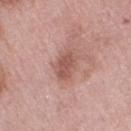| feature | finding |
|---|---|
| notes | catalogued during a skin exam; not biopsied |
| imaging modality | ~15 mm tile from a whole-body skin photo |
| site | the right thigh |
| patient | female, aged around 70 |